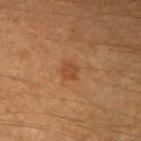Clinical impression:
Part of a total-body skin-imaging series; this lesion was reviewed on a skin check and was not flagged for biopsy.